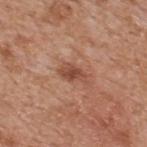Clinical impression:
The lesion was tiled from a total-body skin photograph and was not biopsied.
Clinical summary:
Approximately 3.5 mm at its widest. Imaged with white-light lighting. The lesion is located on the upper back. Automated image analysis of the tile measured border irregularity of about 3 on a 0–10 scale and a color-variation rating of about 3.5/10. The software also gave a nevus-likeness score of about 5/100 and lesion-presence confidence of about 100/100. The patient is a male about 65 years old. A close-up tile cropped from a whole-body skin photograph, about 15 mm across.